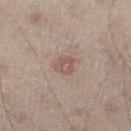follow-up: total-body-photography surveillance lesion; no biopsy
acquisition: ~15 mm crop, total-body skin-cancer survey
illumination: cross-polarized illumination
site: the left thigh
automated lesion analysis: a color-variation rating of about 2.5/10 and peripheral color asymmetry of about 1
size: ≈2.5 mm
patient: male, aged approximately 50